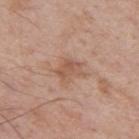biopsy status: total-body-photography surveillance lesion; no biopsy
automated metrics: an outline eccentricity of about 0.7 (0 = round, 1 = elongated)
patient: male, aged around 70
tile lighting: white-light illumination
anatomic site: the left upper arm
image: ~15 mm crop, total-body skin-cancer survey
size: ≈3 mm